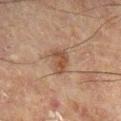– workup — total-body-photography surveillance lesion; no biopsy
– subject — male, aged approximately 60
– acquisition — ~15 mm crop, total-body skin-cancer survey
– tile lighting — cross-polarized illumination
– site — the left leg